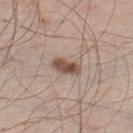Clinical impression: The lesion was photographed on a routine skin check and not biopsied; there is no pathology result. Context: A male subject aged approximately 60. This image is a 15 mm lesion crop taken from a total-body photograph. Located on the leg. This is a white-light tile. Longest diameter approximately 3.5 mm. Automated image analysis of the tile measured a mean CIELAB color near L≈51 a*≈17 b*≈25, roughly 13 lightness units darker than nearby skin, and a normalized lesion–skin contrast near 9.5. The software also gave a border-irregularity rating of about 2/10, internal color variation of about 4.5 on a 0–10 scale, and radial color variation of about 1.5.